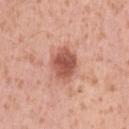| key | value |
|---|---|
| biopsy status | catalogued during a skin exam; not biopsied |
| acquisition | 15 mm crop, total-body photography |
| location | the left upper arm |
| subject | female, in their 40s |